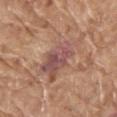Captured during whole-body skin photography for melanoma surveillance; the lesion was not biopsied. An algorithmic analysis of the crop reported a nevus-likeness score of about 0/100 and a detector confidence of about 60 out of 100 that the crop contains a lesion. The patient is a male aged approximately 80. A 15 mm crop from a total-body photograph taken for skin-cancer surveillance. Located on the left upper arm.